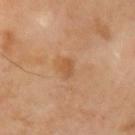Assessment: Recorded during total-body skin imaging; not selected for excision or biopsy. Clinical summary: The lesion's longest dimension is about 2.5 mm. Captured under cross-polarized illumination. From the left upper arm. The subject is a male aged 68 to 72. Cropped from a total-body skin-imaging series; the visible field is about 15 mm.